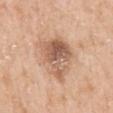Notes:
• follow-up · imaged on a skin check; not biopsied
• patient · female, aged around 40
• lesion diameter · about 5.5 mm
• body site · the mid back
• TBP lesion metrics · radial color variation of about 2.5; an automated nevus-likeness rating near 5 out of 100 and lesion-presence confidence of about 100/100
• acquisition · 15 mm crop, total-body photography